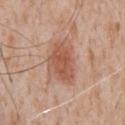Impression: No biopsy was performed on this lesion — it was imaged during a full skin examination and was not determined to be concerning. Acquisition and patient details: Automated tile analysis of the lesion measured a footprint of about 13 mm², a shape eccentricity near 0.8, and a symmetry-axis asymmetry near 0.25. It also reported border irregularity of about 2.5 on a 0–10 scale, internal color variation of about 3 on a 0–10 scale, and radial color variation of about 1. The analysis additionally found a classifier nevus-likeness of about 95/100 and lesion-presence confidence of about 100/100. The lesion is on the chest. A male subject roughly 65 years of age. Imaged with white-light lighting. A close-up tile cropped from a whole-body skin photograph, about 15 mm across.